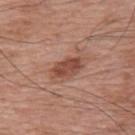No biopsy was performed on this lesion — it was imaged during a full skin examination and was not determined to be concerning. A male patient in their 70s. A 15 mm close-up extracted from a 3D total-body photography capture. This is a white-light tile. On the upper back. Approximately 4 mm at its widest.